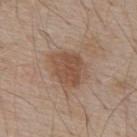Clinical impression:
Recorded during total-body skin imaging; not selected for excision or biopsy.
Clinical summary:
The lesion is located on the upper back. The tile uses white-light illumination. Longest diameter approximately 5 mm. A male patient, aged approximately 55. A lesion tile, about 15 mm wide, cut from a 3D total-body photograph.